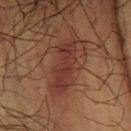Impression: Imaged during a routine full-body skin examination; the lesion was not biopsied and no histopathology is available. Image and clinical context: Longest diameter approximately 7.5 mm. The patient is a male aged 58–62. From the left forearm. Automated image analysis of the tile measured an outline eccentricity of about 0.95 (0 = round, 1 = elongated) and a symmetry-axis asymmetry near 0.35. It also reported a lesion color around L≈27 a*≈18 b*≈21 in CIELAB, a lesion–skin lightness drop of about 6, and a lesion-to-skin contrast of about 7.5 (normalized; higher = more distinct). A roughly 15 mm field-of-view crop from a total-body skin photograph.